A female subject, in their mid-70s. A lesion tile, about 15 mm wide, cut from a 3D total-body photograph. Located on the head or neck — 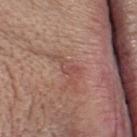On excision, pathology confirmed a cherry hemangioma.